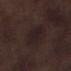Imaged during a routine full-body skin examination; the lesion was not biopsied and no histopathology is available. The lesion's longest dimension is about 4.5 mm. Cropped from a total-body skin-imaging series; the visible field is about 15 mm. On the leg. A male subject aged around 70. Automated image analysis of the tile measured a lesion area of about 13 mm², a shape eccentricity near 0.65, and a symmetry-axis asymmetry near 0.15. It also reported an average lesion color of about L≈19 a*≈11 b*≈11 (CIELAB), a lesion–skin lightness drop of about 4, and a normalized lesion–skin contrast near 7. The analysis additionally found a within-lesion color-variation index near 3/10.